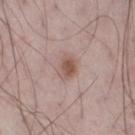notes = no biopsy performed (imaged during a skin exam)
lighting = white-light illumination
size = ~3 mm (longest diameter)
imaging modality = 15 mm crop, total-body photography
automated metrics = a nevus-likeness score of about 95/100 and a lesion-detection confidence of about 100/100
anatomic site = the right thigh
patient = male, aged around 65